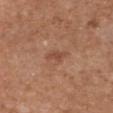patient=female, in their mid- to late 30s; lesion diameter=~2.5 mm (longest diameter); image source=total-body-photography crop, ~15 mm field of view; lighting=white-light illumination; anatomic site=the front of the torso.